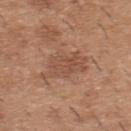biopsy status: catalogued during a skin exam; not biopsied | subject: male, aged 38 to 42 | image: ~15 mm tile from a whole-body skin photo | body site: the upper back | lighting: white-light | automated metrics: an outline eccentricity of about 0.75 (0 = round, 1 = elongated); an average lesion color of about L≈50 a*≈21 b*≈30 (CIELAB), a lesion–skin lightness drop of about 8, and a lesion-to-skin contrast of about 5.5 (normalized; higher = more distinct) | size: ~5 mm (longest diameter).